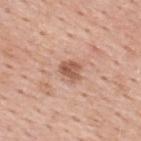The lesion was photographed on a routine skin check and not biopsied; there is no pathology result. The lesion is located on the upper back. A male subject aged around 55. Imaged with white-light lighting. Longest diameter approximately 2.5 mm. A region of skin cropped from a whole-body photographic capture, roughly 15 mm wide.